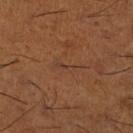notes = total-body-photography surveillance lesion; no biopsy | automated lesion analysis = an area of roughly 3.5 mm², a shape eccentricity near 0.95, and a symmetry-axis asymmetry near 0.5; an average lesion color of about L≈38 a*≈20 b*≈29 (CIELAB), roughly 4 lightness units darker than nearby skin, and a normalized lesion–skin contrast near 4.5; an automated nevus-likeness rating near 0 out of 100 and a detector confidence of about 95 out of 100 that the crop contains a lesion | illumination = cross-polarized illumination | image = 15 mm crop, total-body photography | body site = the right lower leg | patient = male, aged 63–67 | size = ~4 mm (longest diameter).